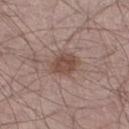biopsy status — no biopsy performed (imaged during a skin exam)
automated lesion analysis — a border-irregularity index near 2/10, internal color variation of about 3 on a 0–10 scale, and a peripheral color-asymmetry measure near 1; an automated nevus-likeness rating near 75 out of 100 and lesion-presence confidence of about 100/100
patient — male, aged 53 to 57
tile lighting — white-light illumination
image — 15 mm crop, total-body photography
lesion size — ~3.5 mm (longest diameter)
body site — the right thigh